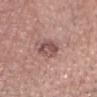From the head or neck.
A 15 mm close-up extracted from a 3D total-body photography capture.
Approximately 3 mm at its widest.
Imaged with white-light lighting.
The patient is a male approximately 40 years of age.
On excision, pathology confirmed an intradermal melanocytic nevus.